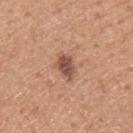{"lighting": "white-light", "lesion_size": {"long_diameter_mm_approx": 3.0}, "site": "arm", "patient": {"sex": "male", "age_approx": 55}, "image": {"source": "total-body photography crop", "field_of_view_mm": 15}}